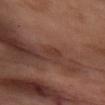Recorded during total-body skin imaging; not selected for excision or biopsy.
On the front of the torso.
A close-up tile cropped from a whole-body skin photograph, about 15 mm across.
The patient is a female in their mid- to late 50s.
Imaged with cross-polarized lighting.